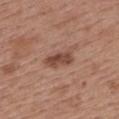biopsy status = catalogued during a skin exam; not biopsied | TBP lesion metrics = a footprint of about 5.5 mm² and an eccentricity of roughly 0.9; a mean CIELAB color near L≈46 a*≈22 b*≈27, roughly 12 lightness units darker than nearby skin, and a normalized lesion–skin contrast near 9; a border-irregularity rating of about 2.5/10 and radial color variation of about 1 | lesion size = ~3.5 mm (longest diameter) | patient = female, approximately 50 years of age | image source = 15 mm crop, total-body photography | site = the upper back | tile lighting = white-light illumination.